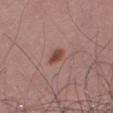workup: imaged on a skin check; not biopsied | lesion size: about 2.5 mm | location: the leg | patient: male, aged 53–57 | automated metrics: an area of roughly 3.5 mm², an eccentricity of roughly 0.8, and a symmetry-axis asymmetry near 0.25; a lesion color around L≈46 a*≈22 b*≈25 in CIELAB, roughly 11 lightness units darker than nearby skin, and a normalized lesion–skin contrast near 8.5; a border-irregularity rating of about 2.5/10, a color-variation rating of about 2.5/10, and a peripheral color-asymmetry measure near 0.5; a classifier nevus-likeness of about 95/100 and lesion-presence confidence of about 100/100 | image: ~15 mm crop, total-body skin-cancer survey.